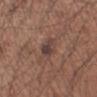biopsy_status: not biopsied; imaged during a skin examination
patient:
  sex: male
  age_approx: 55
automated_metrics:
  eccentricity: 0.45
  shape_asymmetry: 0.2
  cielab_L: 41
  cielab_a: 16
  cielab_b: 20
  vs_skin_contrast_norm: 8.0
  border_irregularity_0_10: 1.5
  color_variation_0_10: 5.5
  peripheral_color_asymmetry: 2.0
  nevus_likeness_0_100: 45
  lesion_detection_confidence_0_100: 100
image:
  source: total-body photography crop
  field_of_view_mm: 15
site: left upper arm
lighting: white-light
lesion_size:
  long_diameter_mm_approx: 2.5